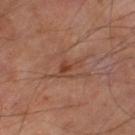{
  "biopsy_status": "not biopsied; imaged during a skin examination",
  "image": {
    "source": "total-body photography crop",
    "field_of_view_mm": 15
  },
  "lesion_size": {
    "long_diameter_mm_approx": 3.5
  },
  "lighting": "cross-polarized",
  "patient": {
    "sex": "male",
    "age_approx": 65
  },
  "site": "leg"
}